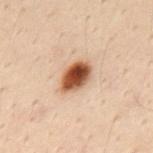This lesion was catalogued during total-body skin photography and was not selected for biopsy.
A male patient, aged 28 to 32.
From the mid back.
This is a cross-polarized tile.
Measured at roughly 4 mm in maximum diameter.
A 15 mm close-up tile from a total-body photography series done for melanoma screening.